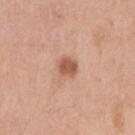Recorded during total-body skin imaging; not selected for excision or biopsy.
A female patient in their mid- to late 50s.
A roughly 15 mm field-of-view crop from a total-body skin photograph.
On the right upper arm.